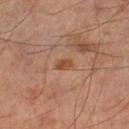Notes:
– workup — no biopsy performed (imaged during a skin exam)
– imaging modality — ~15 mm tile from a whole-body skin photo
– tile lighting — cross-polarized illumination
– automated lesion analysis — a footprint of about 2.5 mm², an outline eccentricity of about 0.85 (0 = round, 1 = elongated), and a symmetry-axis asymmetry near 0.25
– lesion diameter — ~2 mm (longest diameter)
– patient — male, roughly 55 years of age
– anatomic site — the leg